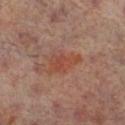Q: Was this lesion biopsied?
A: imaged on a skin check; not biopsied
Q: What lighting was used for the tile?
A: cross-polarized
Q: What is the anatomic site?
A: the right lower leg
Q: What are the patient's age and sex?
A: male, aged around 65
Q: How large is the lesion?
A: ~4 mm (longest diameter)
Q: How was this image acquired?
A: total-body-photography crop, ~15 mm field of view